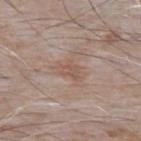{"biopsy_status": "not biopsied; imaged during a skin examination", "patient": {"sex": "male", "age_approx": 65}, "automated_metrics": {"nevus_likeness_0_100": 0}, "image": {"source": "total-body photography crop", "field_of_view_mm": 15}, "lighting": "white-light", "site": "upper back"}